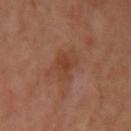biopsy status: imaged on a skin check; not biopsied
body site: the arm
image source: total-body-photography crop, ~15 mm field of view
subject: male, aged around 50
lesion diameter: ~3 mm (longest diameter)
tile lighting: cross-polarized illumination
image-analysis metrics: a lesion–skin lightness drop of about 6 and a lesion-to-skin contrast of about 6 (normalized; higher = more distinct)